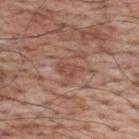Impression: Captured during whole-body skin photography for melanoma surveillance; the lesion was not biopsied. Acquisition and patient details: A male subject aged approximately 70. Measured at roughly 3 mm in maximum diameter. This is a white-light tile. An algorithmic analysis of the crop reported border irregularity of about 4.5 on a 0–10 scale and internal color variation of about 2 on a 0–10 scale. It also reported an automated nevus-likeness rating near 0 out of 100 and a detector confidence of about 95 out of 100 that the crop contains a lesion. Cropped from a total-body skin-imaging series; the visible field is about 15 mm. From the upper back.